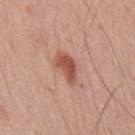Impression: This lesion was catalogued during total-body skin photography and was not selected for biopsy.